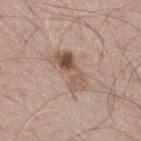Impression:
This lesion was catalogued during total-body skin photography and was not selected for biopsy.
Context:
The lesion is located on the right thigh. Automated tile analysis of the lesion measured a within-lesion color-variation index near 8.5/10 and peripheral color asymmetry of about 3. About 5 mm across. A close-up tile cropped from a whole-body skin photograph, about 15 mm across. The subject is a male in their mid- to late 60s.